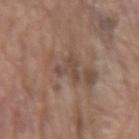notes = imaged on a skin check; not biopsied
lesion diameter = ~3 mm (longest diameter)
acquisition = total-body-photography crop, ~15 mm field of view
patient = male, approximately 80 years of age
anatomic site = the left upper arm
illumination = white-light